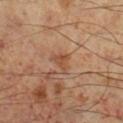Q: Was a biopsy performed?
A: total-body-photography surveillance lesion; no biopsy
Q: How was the tile lit?
A: cross-polarized
Q: Lesion location?
A: the left lower leg
Q: What are the patient's age and sex?
A: male, aged around 60
Q: Automated lesion metrics?
A: a footprint of about 3 mm², an eccentricity of roughly 0.7, and two-axis asymmetry of about 0.5; an average lesion color of about L≈43 a*≈20 b*≈30 (CIELAB), roughly 7 lightness units darker than nearby skin, and a normalized lesion–skin contrast near 6; a border-irregularity index near 5/10, a color-variation rating of about 1/10, and peripheral color asymmetry of about 0; a nevus-likeness score of about 0/100
Q: What is the imaging modality?
A: ~15 mm tile from a whole-body skin photo
Q: Lesion size?
A: ≈2.5 mm